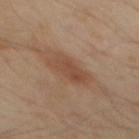The lesion was tiled from a total-body skin photograph and was not biopsied.
A 15 mm close-up extracted from a 3D total-body photography capture.
The patient is a male aged 48–52.
Located on the mid back.